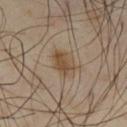notes: no biopsy performed (imaged during a skin exam) | lighting: cross-polarized illumination | patient: male, aged around 55 | TBP lesion metrics: a shape eccentricity near 0.6 and a shape-asymmetry score of about 0.2 (0 = symmetric); border irregularity of about 2 on a 0–10 scale, a color-variation rating of about 3.5/10, and a peripheral color-asymmetry measure near 1; lesion-presence confidence of about 100/100 | location: the left thigh | lesion diameter: ≈3.5 mm | image: total-body-photography crop, ~15 mm field of view.